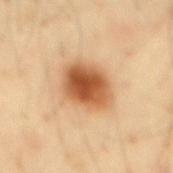Assessment: The lesion was photographed on a routine skin check and not biopsied; there is no pathology result. Image and clinical context: A region of skin cropped from a whole-body photographic capture, roughly 15 mm wide. An algorithmic analysis of the crop reported an eccentricity of roughly 0.5 and two-axis asymmetry of about 0.15. The analysis additionally found roughly 17 lightness units darker than nearby skin and a normalized lesion–skin contrast near 11. It also reported border irregularity of about 1.5 on a 0–10 scale and a within-lesion color-variation index near 6.5/10. The analysis additionally found an automated nevus-likeness rating near 100 out of 100 and lesion-presence confidence of about 100/100. Captured under cross-polarized illumination. The recorded lesion diameter is about 4.5 mm. Located on the mid back. A male subject aged around 40.